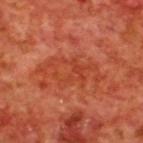This lesion was catalogued during total-body skin photography and was not selected for biopsy. From the upper back. A 15 mm close-up tile from a total-body photography series done for melanoma screening. A male patient, in their 70s.